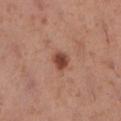An algorithmic analysis of the crop reported a footprint of about 4 mm² and an eccentricity of roughly 0.55. It also reported a border-irregularity rating of about 2/10 and peripheral color asymmetry of about 1. This image is a 15 mm lesion crop taken from a total-body photograph. The lesion is on the right lower leg. A female patient about 40 years old. About 2.5 mm across.